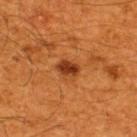notes: imaged on a skin check; not biopsied | lesion diameter: about 3 mm | location: the back | acquisition: total-body-photography crop, ~15 mm field of view | subject: male, aged 58 to 62.